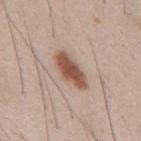Findings:
– follow-up · imaged on a skin check; not biopsied
– lighting · white-light illumination
– lesion size · about 5 mm
– imaging modality · ~15 mm tile from a whole-body skin photo
– patient · male, about 35 years old
– location · the mid back
– automated lesion analysis · a within-lesion color-variation index near 3/10 and peripheral color asymmetry of about 1